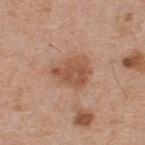{"biopsy_status": "not biopsied; imaged during a skin examination", "automated_metrics": {"area_mm2_approx": 11.0, "eccentricity": 0.55, "shape_asymmetry": 0.35}, "image": {"source": "total-body photography crop", "field_of_view_mm": 15}, "site": "upper back", "lighting": "white-light", "patient": {"sex": "male", "age_approx": 60}, "lesion_size": {"long_diameter_mm_approx": 4.0}}